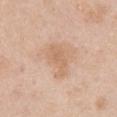<lesion>
  <biopsy_status>not biopsied; imaged during a skin examination</biopsy_status>
  <image>
    <source>total-body photography crop</source>
    <field_of_view_mm>15</field_of_view_mm>
  </image>
  <lighting>white-light</lighting>
  <patient>
    <sex>female</sex>
    <age_approx>60</age_approx>
  </patient>
  <lesion_size>
    <long_diameter_mm_approx>4.5</long_diameter_mm_approx>
  </lesion_size>
  <automated_metrics>
    <area_mm2_approx>8.5</area_mm2_approx>
    <shape_asymmetry>0.4</shape_asymmetry>
  </automated_metrics>
  <site>chest</site>
</lesion>